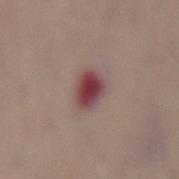biopsy status: total-body-photography surveillance lesion; no biopsy
patient: female, aged 68–72
image: 15 mm crop, total-body photography
illumination: white-light illumination
site: the lower back
size: about 4 mm